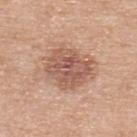Notes:
– subject: male, aged 68 to 72
– location: the upper back
– lesion diameter: ~5.5 mm (longest diameter)
– illumination: white-light
– imaging modality: 15 mm crop, total-body photography
– automated lesion analysis: an area of roughly 19 mm² and an outline eccentricity of about 0.55 (0 = round, 1 = elongated); a mean CIELAB color near L≈56 a*≈21 b*≈28, a lesion–skin lightness drop of about 11, and a lesion-to-skin contrast of about 7.5 (normalized; higher = more distinct)